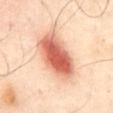workup=total-body-photography surveillance lesion; no biopsy
subject=male, aged approximately 65
site=the chest
image=~15 mm crop, total-body skin-cancer survey